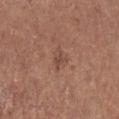The lesion was photographed on a routine skin check and not biopsied; there is no pathology result. Automated tile analysis of the lesion measured a border-irregularity index near 3.5/10, internal color variation of about 1.5 on a 0–10 scale, and radial color variation of about 0.5. About 2.5 mm across. The lesion is on the right lower leg. The tile uses white-light illumination. A 15 mm close-up tile from a total-body photography series done for melanoma screening. A male patient aged 73–77.